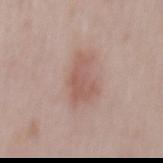This lesion was catalogued during total-body skin photography and was not selected for biopsy. A close-up tile cropped from a whole-body skin photograph, about 15 mm across. The lesion is on the mid back. An algorithmic analysis of the crop reported a lesion area of about 9 mm² and a shape-asymmetry score of about 0.4 (0 = symmetric). The analysis additionally found border irregularity of about 5 on a 0–10 scale, a color-variation rating of about 2/10, and peripheral color asymmetry of about 0.5. The lesion's longest dimension is about 4.5 mm. A male subject in their mid- to late 70s. This is a white-light tile.